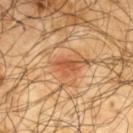<tbp_lesion>
<biopsy_status>not biopsied; imaged during a skin examination</biopsy_status>
<lighting>cross-polarized</lighting>
<automated_metrics>
  <border_irregularity_0_10>3.0</border_irregularity_0_10>
  <color_variation_0_10>2.0</color_variation_0_10>
</automated_metrics>
<image>
  <source>total-body photography crop</source>
  <field_of_view_mm>15</field_of_view_mm>
</image>
<lesion_size>
  <long_diameter_mm_approx>3.0</long_diameter_mm_approx>
</lesion_size>
<site>back</site>
<patient>
  <sex>male</sex>
  <age_approx>65</age_approx>
</patient>
</tbp_lesion>